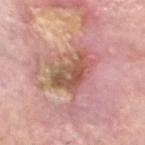From the head or neck.
This is a white-light tile.
A 15 mm crop from a total-body photograph taken for skin-cancer surveillance.
A male patient aged 58 to 62.
The lesion was biopsied, and histopathology showed a squamous cell carcinoma in situ.